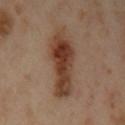illumination = cross-polarized | anatomic site = the arm | subject = female, in their 40s | size = ≈7.5 mm | imaging modality = 15 mm crop, total-body photography.